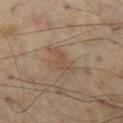follow-up: imaged on a skin check; not biopsied | body site: the left thigh | subject: male, in their mid-50s | lesion diameter: ≈3.5 mm | acquisition: total-body-photography crop, ~15 mm field of view.